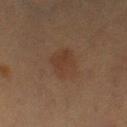notes: total-body-photography surveillance lesion; no biopsy | site: the leg | lesion size: ~4.5 mm (longest diameter) | patient: female, approximately 55 years of age | tile lighting: cross-polarized illumination | image-analysis metrics: a lesion color around L≈31 a*≈15 b*≈23 in CIELAB and a normalized border contrast of about 5 | image source: total-body-photography crop, ~15 mm field of view.